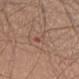This lesion was catalogued during total-body skin photography and was not selected for biopsy. Captured under white-light illumination. Located on the left thigh. An algorithmic analysis of the crop reported an average lesion color of about L≈49 a*≈22 b*≈25 (CIELAB), roughly 7 lightness units darker than nearby skin, and a normalized lesion–skin contrast near 5. The software also gave border irregularity of about 5 on a 0–10 scale, a within-lesion color-variation index near 0/10, and peripheral color asymmetry of about 0. It also reported a lesion-detection confidence of about 95/100. The patient is a male about 65 years old. The lesion's longest dimension is about 3 mm. A 15 mm close-up tile from a total-body photography series done for melanoma screening.